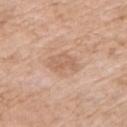This image is a 15 mm lesion crop taken from a total-body photograph. The subject is a female in their mid- to late 70s. Located on the left upper arm.